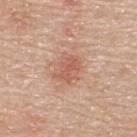Clinical impression:
The lesion was tiled from a total-body skin photograph and was not biopsied.
Image and clinical context:
A roughly 15 mm field-of-view crop from a total-body skin photograph. A male subject, aged around 70. Longest diameter approximately 3.5 mm. The lesion is on the back.